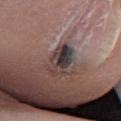lighting: white-light
subject: male, approximately 55 years of age
automated lesion analysis: a lesion color around L≈36 a*≈12 b*≈14 in CIELAB, about 14 CIELAB-L* units darker than the surrounding skin, and a lesion-to-skin contrast of about 12.5 (normalized; higher = more distinct); an automated nevus-likeness rating near 0 out of 100 and lesion-presence confidence of about 55/100
anatomic site: the left lower leg
size: ≈4.5 mm
imaging modality: 15 mm crop, total-body photography